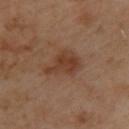biopsy status: catalogued during a skin exam; not biopsied